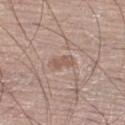- follow-up — total-body-photography surveillance lesion; no biopsy
- site — the left leg
- image — 15 mm crop, total-body photography
- lighting — white-light
- patient — male, aged 78 to 82
- image-analysis metrics — a mean CIELAB color near L≈55 a*≈18 b*≈26, a lesion–skin lightness drop of about 8, and a normalized border contrast of about 6; a nevus-likeness score of about 0/100 and a detector confidence of about 100 out of 100 that the crop contains a lesion